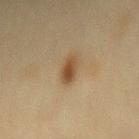Assessment:
Captured during whole-body skin photography for melanoma surveillance; the lesion was not biopsied.
Context:
The lesion-visualizer software estimated a footprint of about 6 mm² and a shape eccentricity near 0.9. A roughly 15 mm field-of-view crop from a total-body skin photograph. From the mid back. About 4 mm across. The tile uses cross-polarized illumination. The subject is a female aged 53–57.